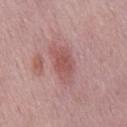{"biopsy_status": "not biopsied; imaged during a skin examination", "lesion_size": {"long_diameter_mm_approx": 4.0}, "automated_metrics": {"area_mm2_approx": 7.0, "shape_asymmetry": 0.2, "cielab_L": 53, "cielab_a": 25, "cielab_b": 23, "vs_skin_darker_L": 9.0, "vs_skin_contrast_norm": 6.5}, "image": {"source": "total-body photography crop", "field_of_view_mm": 15}, "patient": {"sex": "male", "age_approx": 60}, "site": "mid back", "lighting": "white-light"}